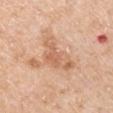* follow-up: catalogued during a skin exam; not biopsied
* lesion diameter: ≈5.5 mm
* illumination: white-light illumination
* subject: male, aged approximately 65
* body site: the left upper arm
* acquisition: ~15 mm tile from a whole-body skin photo